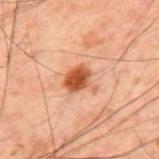<case>
  <biopsy_status>not biopsied; imaged during a skin examination</biopsy_status>
  <automated_metrics>
    <eccentricity>0.4</eccentricity>
    <shape_asymmetry>0.35</shape_asymmetry>
    <cielab_L>42</cielab_L>
    <cielab_a>23</cielab_a>
    <cielab_b>30</cielab_b>
    <vs_skin_darker_L>12.0</vs_skin_darker_L>
    <vs_skin_contrast_norm>10.0</vs_skin_contrast_norm>
    <border_irregularity_0_10>3.5</border_irregularity_0_10>
    <color_variation_0_10>3.5</color_variation_0_10>
    <peripheral_color_asymmetry>1.0</peripheral_color_asymmetry>
    <lesion_detection_confidence_0_100>100</lesion_detection_confidence_0_100>
  </automated_metrics>
  <site>mid back</site>
  <patient>
    <sex>male</sex>
    <age_approx>60</age_approx>
  </patient>
  <image>
    <source>total-body photography crop</source>
    <field_of_view_mm>15</field_of_view_mm>
  </image>
  <lesion_size>
    <long_diameter_mm_approx>3.5</long_diameter_mm_approx>
  </lesion_size>
  <lighting>cross-polarized</lighting>
</case>